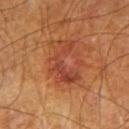Impression: The lesion was photographed on a routine skin check and not biopsied; there is no pathology result. Context: The patient is a male aged 63 to 67. From the left thigh. About 5 mm across. Cropped from a total-body skin-imaging series; the visible field is about 15 mm.